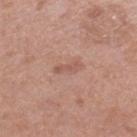Image and clinical context:
About 3 mm across. The tile uses white-light illumination. The lesion is located on the leg. Automated image analysis of the tile measured an area of roughly 3 mm², an eccentricity of roughly 0.95, and two-axis asymmetry of about 0.35. The analysis additionally found a mean CIELAB color near L≈55 a*≈21 b*≈27. And it measured a border-irregularity rating of about 4.5/10, a within-lesion color-variation index near 0/10, and peripheral color asymmetry of about 0. A female patient, in their mid-50s. A region of skin cropped from a whole-body photographic capture, roughly 15 mm wide.